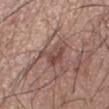Impression:
This lesion was catalogued during total-body skin photography and was not selected for biopsy.